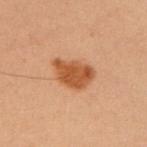Q: Was a biopsy performed?
A: catalogued during a skin exam; not biopsied
Q: What is the imaging modality?
A: 15 mm crop, total-body photography
Q: What are the patient's age and sex?
A: female, approximately 50 years of age
Q: What is the anatomic site?
A: the left upper arm